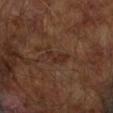On the arm. A 15 mm close-up tile from a total-body photography series done for melanoma screening. Captured under cross-polarized illumination. The patient is a male aged 63–67. About 3.5 mm across.